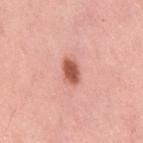The lesion is on the right thigh. Imaged with white-light lighting. A region of skin cropped from a whole-body photographic capture, roughly 15 mm wide. Approximately 3 mm at its widest. The subject is a female roughly 50 years of age.